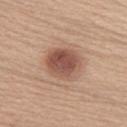Impression: This lesion was catalogued during total-body skin photography and was not selected for biopsy. Clinical summary: Located on the back. Imaged with white-light lighting. A close-up tile cropped from a whole-body skin photograph, about 15 mm across. About 4.5 mm across. A female patient, aged 38–42.